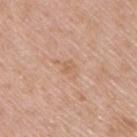Part of a total-body skin-imaging series; this lesion was reviewed on a skin check and was not flagged for biopsy.
Captured under white-light illumination.
A male subject aged 48 to 52.
Located on the upper back.
A lesion tile, about 15 mm wide, cut from a 3D total-body photograph.
The lesion's longest dimension is about 3 mm.